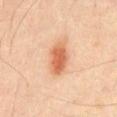Notes:
– follow-up — imaged on a skin check; not biopsied
– imaging modality — 15 mm crop, total-body photography
– anatomic site — the front of the torso
– size — about 4 mm
– illumination — cross-polarized
– automated lesion analysis — a footprint of about 9.5 mm², an outline eccentricity of about 0.8 (0 = round, 1 = elongated), and two-axis asymmetry of about 0.15; a classifier nevus-likeness of about 100/100 and a detector confidence of about 100 out of 100 that the crop contains a lesion
– patient — male, aged approximately 45